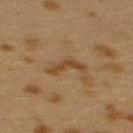Findings:
• biopsy status — no biopsy performed (imaged during a skin exam)
• acquisition — ~15 mm crop, total-body skin-cancer survey
• location — the back
• lighting — cross-polarized
• image-analysis metrics — an average lesion color of about L≈35 a*≈15 b*≈30 (CIELAB) and about 7 CIELAB-L* units darker than the surrounding skin; an automated nevus-likeness rating near 5 out of 100 and a detector confidence of about 95 out of 100 that the crop contains a lesion
• patient — female, about 40 years old
• size — about 4 mm